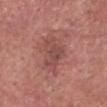Impression:
Part of a total-body skin-imaging series; this lesion was reviewed on a skin check and was not flagged for biopsy.
Acquisition and patient details:
An algorithmic analysis of the crop reported radial color variation of about 1. A male patient, roughly 80 years of age. Located on the head or neck. This is a white-light tile. Approximately 5 mm at its widest. A 15 mm close-up extracted from a 3D total-body photography capture.